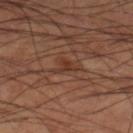The lesion was tiled from a total-body skin photograph and was not biopsied. This is a cross-polarized tile. Cropped from a whole-body photographic skin survey; the tile spans about 15 mm. Longest diameter approximately 3.5 mm. A male patient, aged approximately 60. From the right lower leg.